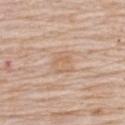{
  "biopsy_status": "not biopsied; imaged during a skin examination",
  "site": "upper back",
  "lighting": "white-light",
  "image": {
    "source": "total-body photography crop",
    "field_of_view_mm": 15
  },
  "patient": {
    "sex": "female",
    "age_approx": 70
  }
}